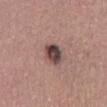  biopsy_status: not biopsied; imaged during a skin examination
  site: back
  image:
    source: total-body photography crop
    field_of_view_mm: 15
  patient:
    sex: male
    age_approx: 40